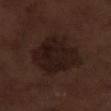Case summary:
• notes — imaged on a skin check; not biopsied
• location — the right lower leg
• lesion size — about 6 mm
• automated metrics — an area of roughly 26 mm² and an outline eccentricity of about 0.3 (0 = round, 1 = elongated)
• image — 15 mm crop, total-body photography
• illumination — white-light
• patient — male, aged 68 to 72